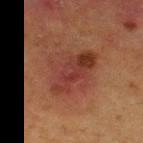Captured during whole-body skin photography for melanoma surveillance; the lesion was not biopsied. A region of skin cropped from a whole-body photographic capture, roughly 15 mm wide. The patient is a female approximately 50 years of age. Located on the right thigh. This is a cross-polarized tile.